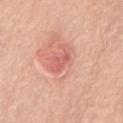No biopsy was performed on this lesion — it was imaged during a full skin examination and was not determined to be concerning.
Approximately 3 mm at its widest.
A male patient, approximately 80 years of age.
On the front of the torso.
A 15 mm close-up extracted from a 3D total-body photography capture.